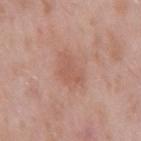The lesion was photographed on a routine skin check and not biopsied; there is no pathology result.
The lesion is on the back.
A close-up tile cropped from a whole-body skin photograph, about 15 mm across.
A male subject aged 53 to 57.